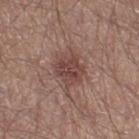Part of a total-body skin-imaging series; this lesion was reviewed on a skin check and was not flagged for biopsy. The tile uses white-light illumination. A 15 mm crop from a total-body photograph taken for skin-cancer surveillance. A male subject approximately 40 years of age. On the right thigh.